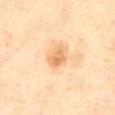Findings:
– notes · no biopsy performed (imaged during a skin exam)
– image · ~15 mm tile from a whole-body skin photo
– patient · male, in their 70s
– lesion size · about 3 mm
– tile lighting · cross-polarized illumination
– site · the abdomen